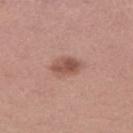Assessment:
Part of a total-body skin-imaging series; this lesion was reviewed on a skin check and was not flagged for biopsy.
Image and clinical context:
Imaged with white-light lighting. A lesion tile, about 15 mm wide, cut from a 3D total-body photograph. A female patient aged approximately 30. Automated tile analysis of the lesion measured a mean CIELAB color near L≈52 a*≈22 b*≈26, a lesion–skin lightness drop of about 11, and a normalized border contrast of about 7.5. The software also gave a border-irregularity index near 2/10, a within-lesion color-variation index near 4/10, and peripheral color asymmetry of about 1.5. The analysis additionally found an automated nevus-likeness rating near 95 out of 100 and a lesion-detection confidence of about 100/100. The lesion is on the left thigh. The lesion's longest dimension is about 3.5 mm.